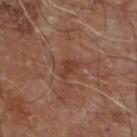Impression:
This lesion was catalogued during total-body skin photography and was not selected for biopsy.
Image and clinical context:
Cropped from a total-body skin-imaging series; the visible field is about 15 mm. An algorithmic analysis of the crop reported a lesion area of about 3.5 mm², a shape eccentricity near 0.8, and a shape-asymmetry score of about 0.4 (0 = symmetric). The analysis additionally found border irregularity of about 4 on a 0–10 scale. It also reported an automated nevus-likeness rating near 0 out of 100 and lesion-presence confidence of about 100/100. From the leg. The tile uses cross-polarized illumination. The recorded lesion diameter is about 2.5 mm. A male patient, aged approximately 70.